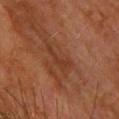Assessment:
The lesion was tiled from a total-body skin photograph and was not biopsied.
Acquisition and patient details:
The lesion is on the upper back. The subject is a female in their mid-50s. This is a cross-polarized tile. A roughly 15 mm field-of-view crop from a total-body skin photograph.